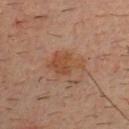Assessment:
This lesion was catalogued during total-body skin photography and was not selected for biopsy.
Context:
A male subject about 35 years old. A lesion tile, about 15 mm wide, cut from a 3D total-body photograph. Captured under cross-polarized illumination. Longest diameter approximately 4.5 mm. Located on the chest.